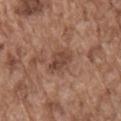{
  "biopsy_status": "not biopsied; imaged during a skin examination",
  "site": "chest",
  "lesion_size": {
    "long_diameter_mm_approx": 3.0
  },
  "patient": {
    "sex": "male",
    "age_approx": 75
  },
  "image": {
    "source": "total-body photography crop",
    "field_of_view_mm": 15
  },
  "lighting": "white-light"
}